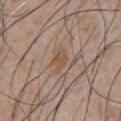No biopsy was performed on this lesion — it was imaged during a full skin examination and was not determined to be concerning. A 15 mm close-up tile from a total-body photography series done for melanoma screening. A male patient in their 50s. Approximately 2.5 mm at its widest. On the chest.